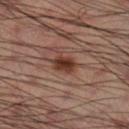Imaged during a routine full-body skin examination; the lesion was not biopsied and no histopathology is available. A male subject aged approximately 50. Approximately 3.5 mm at its widest. Located on the leg. A roughly 15 mm field-of-view crop from a total-body skin photograph.